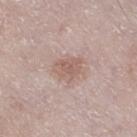workup: total-body-photography surveillance lesion; no biopsy
illumination: white-light
patient: male, approximately 65 years of age
image source: 15 mm crop, total-body photography
lesion diameter: ≈3.5 mm
location: the right thigh